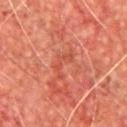| field | value |
|---|---|
| notes | total-body-photography surveillance lesion; no biopsy |
| diameter | ≈4 mm |
| body site | the front of the torso |
| subject | male, roughly 65 years of age |
| image source | ~15 mm tile from a whole-body skin photo |
| illumination | cross-polarized |